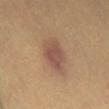Clinical impression:
Captured during whole-body skin photography for melanoma surveillance; the lesion was not biopsied.
Background:
On the lower back. Approximately 5 mm at its widest. An algorithmic analysis of the crop reported roughly 9 lightness units darker than nearby skin and a normalized lesion–skin contrast near 8. And it measured a nevus-likeness score of about 80/100 and a detector confidence of about 100 out of 100 that the crop contains a lesion. Imaged with cross-polarized lighting. A 15 mm close-up tile from a total-body photography series done for melanoma screening. A patient aged 58 to 62.